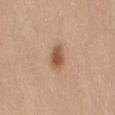Imaged during a routine full-body skin examination; the lesion was not biopsied and no histopathology is available. Cropped from a whole-body photographic skin survey; the tile spans about 15 mm. On the lower back. The subject is a female about 55 years old.